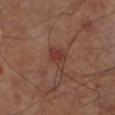biopsy status: total-body-photography surveillance lesion; no biopsy | automated lesion analysis: an eccentricity of roughly 0.65 and a shape-asymmetry score of about 0.25 (0 = symmetric); a lesion color around L≈35 a*≈22 b*≈23 in CIELAB and a normalized lesion–skin contrast near 6; a border-irregularity index near 2.5/10, a within-lesion color-variation index near 3/10, and peripheral color asymmetry of about 1 | location: the left lower leg | subject: male, in their 70s | image source: ~15 mm tile from a whole-body skin photo.